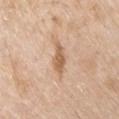Clinical impression: Part of a total-body skin-imaging series; this lesion was reviewed on a skin check and was not flagged for biopsy. Acquisition and patient details: The lesion's longest dimension is about 4 mm. The patient is a male roughly 80 years of age. Located on the chest. Automated image analysis of the tile measured a footprint of about 5 mm² and an outline eccentricity of about 0.9 (0 = round, 1 = elongated). And it measured a lesion-detection confidence of about 100/100. A roughly 15 mm field-of-view crop from a total-body skin photograph.